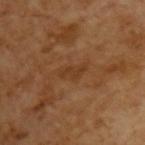Q: Was a biopsy performed?
A: total-body-photography surveillance lesion; no biopsy
Q: Lesion size?
A: about 3 mm
Q: How was the tile lit?
A: cross-polarized
Q: What are the patient's age and sex?
A: male, in their mid-60s
Q: What kind of image is this?
A: ~15 mm crop, total-body skin-cancer survey